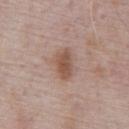Captured during whole-body skin photography for melanoma surveillance; the lesion was not biopsied. About 4 mm across. This is a white-light tile. The lesion-visualizer software estimated an eccentricity of roughly 0.85. The patient is a male about 70 years old. A 15 mm close-up extracted from a 3D total-body photography capture. From the abdomen.